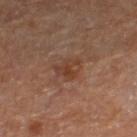Captured during whole-body skin photography for melanoma surveillance; the lesion was not biopsied.